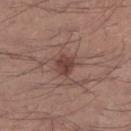Imaged during a routine full-body skin examination; the lesion was not biopsied and no histopathology is available. The tile uses white-light illumination. A male subject about 40 years old. A 15 mm close-up extracted from a 3D total-body photography capture. The lesion is located on the leg. Approximately 3 mm at its widest. Automated tile analysis of the lesion measured a lesion area of about 5 mm², an outline eccentricity of about 0.5 (0 = round, 1 = elongated), and a symmetry-axis asymmetry near 0.3. And it measured an average lesion color of about L≈42 a*≈20 b*≈23 (CIELAB) and a normalized lesion–skin contrast near 8.